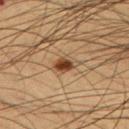Clinical impression:
Imaged during a routine full-body skin examination; the lesion was not biopsied and no histopathology is available.
Background:
The lesion is on the arm. The tile uses cross-polarized illumination. The subject is a male aged 53–57. A lesion tile, about 15 mm wide, cut from a 3D total-body photograph.